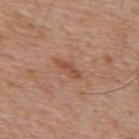Findings:
• follow-up · no biopsy performed (imaged during a skin exam)
• tile lighting · white-light
• patient · male, in their 60s
• image · total-body-photography crop, ~15 mm field of view
• lesion diameter · about 3.5 mm
• body site · the mid back